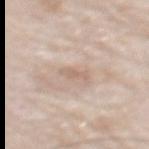follow-up = catalogued during a skin exam; not biopsied | subject = male, aged around 75 | automated lesion analysis = a lesion–skin lightness drop of about 7 and a normalized lesion–skin contrast near 5 | lesion diameter = about 2.5 mm | body site = the mid back | lighting = white-light illumination | acquisition = total-body-photography crop, ~15 mm field of view.